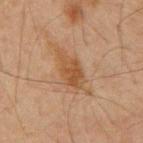• notes — imaged on a skin check; not biopsied
• subject — male, about 65 years old
• image source — ~15 mm tile from a whole-body skin photo
• lighting — cross-polarized illumination
• lesion size — ≈6.5 mm
• TBP lesion metrics — a footprint of about 12 mm² and a symmetry-axis asymmetry near 0.35; a mean CIELAB color near L≈42 a*≈16 b*≈30, a lesion–skin lightness drop of about 7, and a normalized lesion–skin contrast near 7; a nevus-likeness score of about 45/100 and a detector confidence of about 100 out of 100 that the crop contains a lesion
• location — the mid back